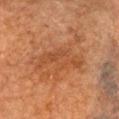workup — imaged on a skin check; not biopsied
subject — female, aged 68–72
diameter — about 6 mm
image — 15 mm crop, total-body photography
tile lighting — cross-polarized
body site — the head or neck